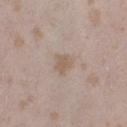TBP lesion metrics: a lesion area of about 4.5 mm², a shape eccentricity near 0.4, and a shape-asymmetry score of about 0.3 (0 = symmetric); a border-irregularity rating of about 3/10, internal color variation of about 1.5 on a 0–10 scale, and a peripheral color-asymmetry measure near 0.5
illumination: white-light illumination
lesion diameter: ~2.5 mm (longest diameter)
imaging modality: ~15 mm tile from a whole-body skin photo
body site: the left thigh
subject: female, approximately 25 years of age